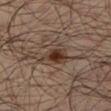This lesion was catalogued during total-body skin photography and was not selected for biopsy.
Imaged with cross-polarized lighting.
The lesion is on the left lower leg.
About 4 mm across.
A male subject about 35 years old.
A close-up tile cropped from a whole-body skin photograph, about 15 mm across.